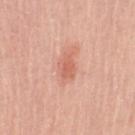{"biopsy_status": "not biopsied; imaged during a skin examination", "image": {"source": "total-body photography crop", "field_of_view_mm": 15}, "lesion_size": {"long_diameter_mm_approx": 3.0}, "site": "arm", "patient": {"sex": "female", "age_approx": 65}}